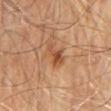<tbp_lesion>
<biopsy_status>not biopsied; imaged during a skin examination</biopsy_status>
<lesion_size>
  <long_diameter_mm_approx>3.5</long_diameter_mm_approx>
</lesion_size>
<image>
  <source>total-body photography crop</source>
  <field_of_view_mm>15</field_of_view_mm>
</image>
<lighting>cross-polarized</lighting>
<automated_metrics>
  <cielab_L>50</cielab_L>
  <cielab_a>24</cielab_a>
  <cielab_b>35</cielab_b>
  <vs_skin_darker_L>9.0</vs_skin_darker_L>
  <vs_skin_contrast_norm>7.0</vs_skin_contrast_norm>
  <nevus_likeness_0_100>50</nevus_likeness_0_100>
  <lesion_detection_confidence_0_100>100</lesion_detection_confidence_0_100>
</automated_metrics>
<patient>
  <sex>male</sex>
  <age_approx>70</age_approx>
</patient>
<site>mid back</site>
</tbp_lesion>